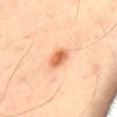Part of a total-body skin-imaging series; this lesion was reviewed on a skin check and was not flagged for biopsy.
Measured at roughly 3 mm in maximum diameter.
This is a cross-polarized tile.
A male patient aged around 50.
A close-up tile cropped from a whole-body skin photograph, about 15 mm across.
The lesion is on the leg.